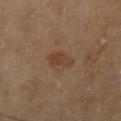{
  "biopsy_status": "not biopsied; imaged during a skin examination",
  "automated_metrics": {
    "area_mm2_approx": 5.0,
    "eccentricity": 0.85,
    "shape_asymmetry": 0.25
  },
  "image": {
    "source": "total-body photography crop",
    "field_of_view_mm": 15
  },
  "site": "right lower leg",
  "lighting": "cross-polarized",
  "patient": {
    "sex": "male",
    "age_approx": 65
  },
  "lesion_size": {
    "long_diameter_mm_approx": 3.5
  }
}